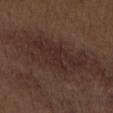Imaged during a routine full-body skin examination; the lesion was not biopsied and no histopathology is available. A lesion tile, about 15 mm wide, cut from a 3D total-body photograph. The patient is a male aged 68 to 72. The lesion is on the left upper arm.